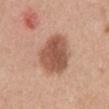Background:
Imaged with white-light lighting. A female patient, roughly 40 years of age. A lesion tile, about 15 mm wide, cut from a 3D total-body photograph. About 6 mm across. From the front of the torso.